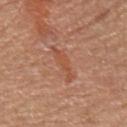<lesion>
<biopsy_status>not biopsied; imaged during a skin examination</biopsy_status>
<site>arm</site>
<image>
  <source>total-body photography crop</source>
  <field_of_view_mm>15</field_of_view_mm>
</image>
<automated_metrics>
  <cielab_L>51</cielab_L>
  <cielab_a>26</cielab_a>
  <cielab_b>33</cielab_b>
  <vs_skin_contrast_norm>5.5</vs_skin_contrast_norm>
</automated_metrics>
<lighting>white-light</lighting>
<patient>
  <sex>female</sex>
  <age_approx>60</age_approx>
</patient>
</lesion>